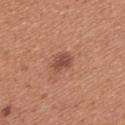– follow-up: no biopsy performed (imaged during a skin exam)